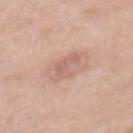Imaged during a routine full-body skin examination; the lesion was not biopsied and no histopathology is available.
The recorded lesion diameter is about 4.5 mm.
Cropped from a whole-body photographic skin survey; the tile spans about 15 mm.
Imaged with white-light lighting.
Located on the mid back.
A female patient, approximately 40 years of age.
Automated tile analysis of the lesion measured a footprint of about 6.5 mm², an outline eccentricity of about 0.9 (0 = round, 1 = elongated), and a shape-asymmetry score of about 0.4 (0 = symmetric). And it measured a mean CIELAB color near L≈62 a*≈21 b*≈26 and roughly 8 lightness units darker than nearby skin. And it measured a border-irregularity index near 5.5/10, internal color variation of about 2 on a 0–10 scale, and a peripheral color-asymmetry measure near 0.5.